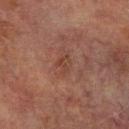  biopsy_status: not biopsied; imaged during a skin examination
  site: right lower leg
  image:
    source: total-body photography crop
    field_of_view_mm: 15
  patient:
    sex: male
    age_approx: 75